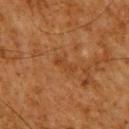Clinical impression:
Recorded during total-body skin imaging; not selected for excision or biopsy.
Acquisition and patient details:
A male patient, aged 58–62. Located on the upper back. A 15 mm close-up tile from a total-body photography series done for melanoma screening. The tile uses cross-polarized illumination. Automated image analysis of the tile measured border irregularity of about 4 on a 0–10 scale, internal color variation of about 0.5 on a 0–10 scale, and peripheral color asymmetry of about 0. It also reported a nevus-likeness score of about 0/100.